The lesion is on the mid back · a roughly 15 mm field-of-view crop from a total-body skin photograph · a female subject, in their 50s: 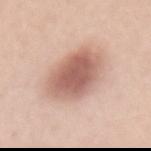Histopathologically confirmed as a dysplastic (Clark) nevus.